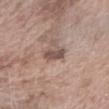Clinical impression:
The lesion was photographed on a routine skin check and not biopsied; there is no pathology result.
Background:
Longest diameter approximately 2.5 mm. A 15 mm close-up tile from a total-body photography series done for melanoma screening. The subject is a female roughly 75 years of age. Automated tile analysis of the lesion measured an average lesion color of about L≈50 a*≈17 b*≈22 (CIELAB) and a lesion-to-skin contrast of about 8.5 (normalized; higher = more distinct). The software also gave a border-irregularity rating of about 3/10, a within-lesion color-variation index near 2/10, and peripheral color asymmetry of about 0.5. The analysis additionally found a classifier nevus-likeness of about 5/100. The lesion is on the right forearm. The tile uses white-light illumination.